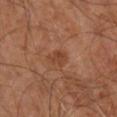Part of a total-body skin-imaging series; this lesion was reviewed on a skin check and was not flagged for biopsy.
Captured under cross-polarized illumination.
On the right leg.
The lesion's longest dimension is about 2.5 mm.
A 15 mm close-up extracted from a 3D total-body photography capture.
A male subject, aged 58–62.